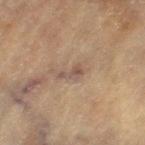Q: Is there a histopathology result?
A: no biopsy performed (imaged during a skin exam)
Q: Lesion size?
A: ≈3 mm
Q: Lesion location?
A: the left thigh
Q: What did automated image analysis measure?
A: a lesion color around L≈43 a*≈14 b*≈22 in CIELAB and about 7 CIELAB-L* units darker than the surrounding skin
Q: Illumination type?
A: cross-polarized illumination
Q: Patient demographics?
A: female, aged approximately 80
Q: How was this image acquired?
A: 15 mm crop, total-body photography